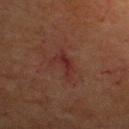Image and clinical context: A 15 mm close-up extracted from a 3D total-body photography capture. The lesion is located on the head or neck. A female subject, aged 63 to 67.